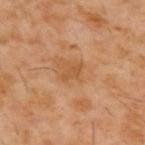Part of a total-body skin-imaging series; this lesion was reviewed on a skin check and was not flagged for biopsy.
A male subject aged 58 to 62.
Cropped from a total-body skin-imaging series; the visible field is about 15 mm.
Automated tile analysis of the lesion measured an average lesion color of about L≈49 a*≈21 b*≈37 (CIELAB), about 7 CIELAB-L* units darker than the surrounding skin, and a normalized border contrast of about 6. It also reported a color-variation rating of about 0/10 and radial color variation of about 0.
The lesion is on the upper back.
This is a cross-polarized tile.
About 2.5 mm across.